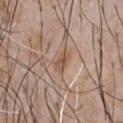Clinical impression:
Part of a total-body skin-imaging series; this lesion was reviewed on a skin check and was not flagged for biopsy.
Context:
This is a white-light tile. A male patient, in their 50s. From the front of the torso. A lesion tile, about 15 mm wide, cut from a 3D total-body photograph. The lesion-visualizer software estimated an eccentricity of roughly 0.85 and two-axis asymmetry of about 0.3. The analysis additionally found roughly 9 lightness units darker than nearby skin and a lesion-to-skin contrast of about 7.5 (normalized; higher = more distinct). It also reported border irregularity of about 3 on a 0–10 scale, internal color variation of about 2.5 on a 0–10 scale, and a peripheral color-asymmetry measure near 1. Approximately 2.5 mm at its widest.